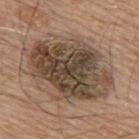Case summary:
– biopsy status: catalogued during a skin exam; not biopsied
– subject: male, in their mid- to late 60s
– image source: 15 mm crop, total-body photography
– location: the upper back
– diameter: about 10 mm